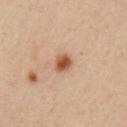<case>
  <biopsy_status>not biopsied; imaged during a skin examination</biopsy_status>
  <image>
    <source>total-body photography crop</source>
    <field_of_view_mm>15</field_of_view_mm>
  </image>
  <patient>
    <sex>male</sex>
    <age_approx>40</age_approx>
  </patient>
  <site>left upper arm</site>
  <lighting>cross-polarized</lighting>
  <lesion_size>
    <long_diameter_mm_approx>2.0</long_diameter_mm_approx>
  </lesion_size>
</case>